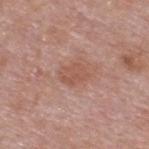Imaged during a routine full-body skin examination; the lesion was not biopsied and no histopathology is available. A 15 mm crop from a total-body photograph taken for skin-cancer surveillance. A male patient, aged around 55. On the upper back.